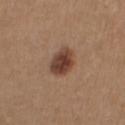Part of a total-body skin-imaging series; this lesion was reviewed on a skin check and was not flagged for biopsy. A 15 mm close-up extracted from a 3D total-body photography capture. This is a white-light tile. A female subject, in their 40s. About 3.5 mm across. The lesion is located on the arm.